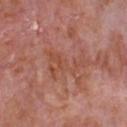biopsy status: catalogued during a skin exam; not biopsied
diameter: ~5.5 mm (longest diameter)
body site: the chest
TBP lesion metrics: a lesion area of about 10 mm², a shape eccentricity near 0.8, and two-axis asymmetry of about 0.7; an average lesion color of about L≈50 a*≈26 b*≈30 (CIELAB), roughly 6 lightness units darker than nearby skin, and a normalized lesion–skin contrast near 5.5; an automated nevus-likeness rating near 0 out of 100 and lesion-presence confidence of about 95/100
lighting: white-light
subject: male, approximately 65 years of age
acquisition: ~15 mm tile from a whole-body skin photo